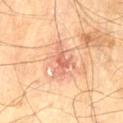<tbp_lesion>
  <biopsy_status>not biopsied; imaged during a skin examination</biopsy_status>
  <site>left thigh</site>
  <lighting>cross-polarized</lighting>
  <patient>
    <sex>male</sex>
    <age_approx>70</age_approx>
  </patient>
  <image>
    <source>total-body photography crop</source>
    <field_of_view_mm>15</field_of_view_mm>
  </image>
  <lesion_size>
    <long_diameter_mm_approx>4.5</long_diameter_mm_approx>
  </lesion_size>
</tbp_lesion>